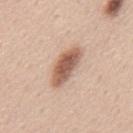{
  "biopsy_status": "not biopsied; imaged during a skin examination",
  "lesion_size": {
    "long_diameter_mm_approx": 5.0
  },
  "image": {
    "source": "total-body photography crop",
    "field_of_view_mm": 15
  },
  "site": "mid back",
  "lighting": "white-light",
  "automated_metrics": {
    "area_mm2_approx": 10.0,
    "eccentricity": 0.9,
    "shape_asymmetry": 0.15,
    "border_irregularity_0_10": 2.5,
    "peripheral_color_asymmetry": 1.0,
    "nevus_likeness_0_100": 95,
    "lesion_detection_confidence_0_100": 100
  },
  "patient": {
    "sex": "male",
    "age_approx": 45
  }
}